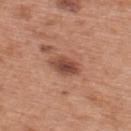Captured during whole-body skin photography for melanoma surveillance; the lesion was not biopsied.
A 15 mm crop from a total-body photograph taken for skin-cancer surveillance.
The tile uses white-light illumination.
The lesion is on the upper back.
The subject is a male in their 70s.